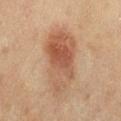{"biopsy_status": "not biopsied; imaged during a skin examination", "image": {"source": "total-body photography crop", "field_of_view_mm": 15}, "lesion_size": {"long_diameter_mm_approx": 7.5}, "patient": {"sex": "female", "age_approx": 55}, "lighting": "cross-polarized", "automated_metrics": {"color_variation_0_10": 6.5, "peripheral_color_asymmetry": 2.0, "lesion_detection_confidence_0_100": 100}, "site": "left thigh"}